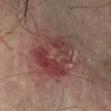Impression:
Captured during whole-body skin photography for melanoma surveillance; the lesion was not biopsied.
Context:
A lesion tile, about 15 mm wide, cut from a 3D total-body photograph. The lesion is located on the right lower leg. A male subject, in their mid-70s.